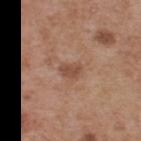A male patient, aged approximately 55. On the back. Cropped from a whole-body photographic skin survey; the tile spans about 15 mm.